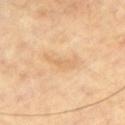biopsy status=catalogued during a skin exam; not biopsied | image=~15 mm crop, total-body skin-cancer survey | body site=the left upper arm | patient=male, aged around 60.